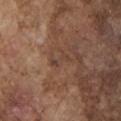Q: Was this lesion biopsied?
A: imaged on a skin check; not biopsied
Q: What lighting was used for the tile?
A: white-light
Q: Automated lesion metrics?
A: an average lesion color of about L≈41 a*≈17 b*≈25 (CIELAB) and roughly 6 lightness units darker than nearby skin; a classifier nevus-likeness of about 0/100 and a detector confidence of about 75 out of 100 that the crop contains a lesion
Q: Lesion location?
A: the left upper arm
Q: What is the imaging modality?
A: ~15 mm crop, total-body skin-cancer survey
Q: What is the lesion's diameter?
A: about 2.5 mm
Q: Patient demographics?
A: male, aged approximately 75